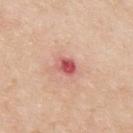illumination: white-light | automated lesion analysis: an area of roughly 4 mm², a shape eccentricity near 0.65, and a symmetry-axis asymmetry near 0.15; a mean CIELAB color near L≈56 a*≈33 b*≈27 and a lesion-to-skin contrast of about 9.5 (normalized; higher = more distinct) | acquisition: 15 mm crop, total-body photography | body site: the upper back | lesion size: ≈2.5 mm | patient: male, aged 33–37.